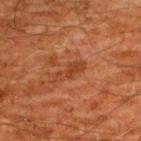{
  "biopsy_status": "not biopsied; imaged during a skin examination",
  "site": "back",
  "lesion_size": {
    "long_diameter_mm_approx": 3.5
  },
  "lighting": "cross-polarized",
  "image": {
    "source": "total-body photography crop",
    "field_of_view_mm": 15
  },
  "patient": {
    "sex": "male",
    "age_approx": 60
  }
}